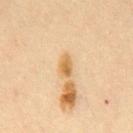<case>
<biopsy_status>not biopsied; imaged during a skin examination</biopsy_status>
<lighting>cross-polarized</lighting>
<patient>
  <sex>male</sex>
  <age_approx>35</age_approx>
</patient>
<site>chest</site>
<lesion_size>
  <long_diameter_mm_approx>2.5</long_diameter_mm_approx>
</lesion_size>
<image>
  <source>total-body photography crop</source>
  <field_of_view_mm>15</field_of_view_mm>
</image>
</case>